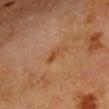notes = total-body-photography surveillance lesion; no biopsy
lesion size = ≈2.5 mm
image source = ~15 mm crop, total-body skin-cancer survey
image-analysis metrics = an area of roughly 2.5 mm² and a shape eccentricity near 0.9; a border-irregularity rating of about 3.5/10 and radial color variation of about 0; lesion-presence confidence of about 100/100
subject = female, approximately 50 years of age
anatomic site = the chest
illumination = cross-polarized illumination